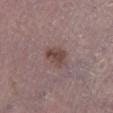| feature | finding |
|---|---|
| biopsy status | imaged on a skin check; not biopsied |
| site | the leg |
| lesion size | ≈3 mm |
| acquisition | 15 mm crop, total-body photography |
| patient | male, aged 68–72 |